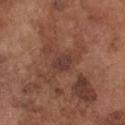The lesion was tiled from a total-body skin photograph and was not biopsied.
The recorded lesion diameter is about 2.5 mm.
A male subject roughly 75 years of age.
Cropped from a total-body skin-imaging series; the visible field is about 15 mm.
Located on the front of the torso.
This is a white-light tile.